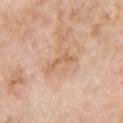follow-up — catalogued during a skin exam; not biopsied
patient — male, aged 58–62
site — the front of the torso
image source — ~15 mm tile from a whole-body skin photo